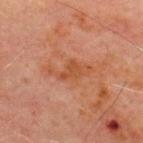biopsy status=total-body-photography surveillance lesion; no biopsy
body site=the chest
image=total-body-photography crop, ~15 mm field of view
patient=male, aged 68–72
lesion diameter=~5 mm (longest diameter)
automated lesion analysis=a border-irregularity index near 5.5/10 and a within-lesion color-variation index near 2/10; a detector confidence of about 100 out of 100 that the crop contains a lesion